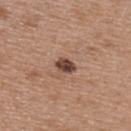Recorded during total-body skin imaging; not selected for excision or biopsy. On the back. A region of skin cropped from a whole-body photographic capture, roughly 15 mm wide. A female patient in their 40s.